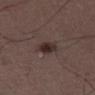<lesion>
<biopsy_status>not biopsied; imaged during a skin examination</biopsy_status>
<patient>
  <sex>male</sex>
  <age_approx>50</age_approx>
</patient>
<site>right thigh</site>
<lighting>white-light</lighting>
<lesion_size>
  <long_diameter_mm_approx>3.5</long_diameter_mm_approx>
</lesion_size>
<image>
  <source>total-body photography crop</source>
  <field_of_view_mm>15</field_of_view_mm>
</image>
<automated_metrics>
  <area_mm2_approx>5.5</area_mm2_approx>
  <eccentricity>0.85</eccentricity>
  <shape_asymmetry>0.25</shape_asymmetry>
  <border_irregularity_0_10>2.5</border_irregularity_0_10>
  <color_variation_0_10>5.0</color_variation_0_10>
  <peripheral_color_asymmetry>1.5</peripheral_color_asymmetry>
</automated_metrics>
</lesion>